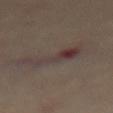Impression: Part of a total-body skin-imaging series; this lesion was reviewed on a skin check and was not flagged for biopsy. Image and clinical context: A female subject aged approximately 60. The lesion is on the abdomen. The lesion's longest dimension is about 5 mm. The total-body-photography lesion software estimated a border-irregularity rating of about 7.5/10 and a color-variation rating of about 6.5/10. Cropped from a whole-body photographic skin survey; the tile spans about 15 mm. Imaged with cross-polarized lighting.